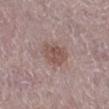follow-up: imaged on a skin check; not biopsied
subject: female, in their mid- to late 60s
location: the right lower leg
imaging modality: 15 mm crop, total-body photography
tile lighting: white-light illumination